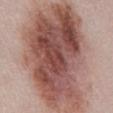Q: Is there a histopathology result?
A: imaged on a skin check; not biopsied
Q: How was the tile lit?
A: white-light illumination
Q: How was this image acquired?
A: total-body-photography crop, ~15 mm field of view
Q: What is the anatomic site?
A: the abdomen
Q: Who is the patient?
A: female, in their 50s
Q: Automated lesion metrics?
A: a footprint of about 105 mm², an eccentricity of roughly 0.8, and a symmetry-axis asymmetry near 0.2; a lesion color around L≈52 a*≈21 b*≈24 in CIELAB, roughly 16 lightness units darker than nearby skin, and a normalized border contrast of about 11; a detector confidence of about 100 out of 100 that the crop contains a lesion